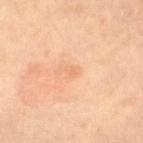Q: Patient demographics?
A: female, in their 70s
Q: What is the anatomic site?
A: the left thigh
Q: What is the imaging modality?
A: total-body-photography crop, ~15 mm field of view